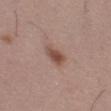<case>
<patient>
  <sex>female</sex>
  <age_approx>50</age_approx>
</patient>
<lighting>white-light</lighting>
<image>
  <source>total-body photography crop</source>
  <field_of_view_mm>15</field_of_view_mm>
</image>
<site>leg</site>
<lesion_size>
  <long_diameter_mm_approx>3.0</long_diameter_mm_approx>
</lesion_size>
</case>